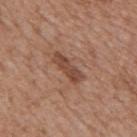Imaged during a routine full-body skin examination; the lesion was not biopsied and no histopathology is available. A male patient in their mid-60s. A 15 mm close-up extracted from a 3D total-body photography capture. From the back. Automated image analysis of the tile measured a lesion area of about 6 mm², a shape eccentricity near 0.95, and a symmetry-axis asymmetry near 0.25.